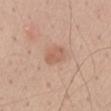{"biopsy_status": "not biopsied; imaged during a skin examination", "site": "chest", "patient": {"sex": "male", "age_approx": 60}, "lesion_size": {"long_diameter_mm_approx": 3.0}, "image": {"source": "total-body photography crop", "field_of_view_mm": 15}}